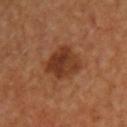This lesion was catalogued during total-body skin photography and was not selected for biopsy.